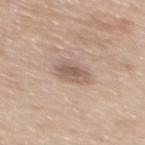Impression:
The lesion was photographed on a routine skin check and not biopsied; there is no pathology result.
Background:
Imaged with white-light lighting. Cropped from a total-body skin-imaging series; the visible field is about 15 mm. The lesion-visualizer software estimated a lesion area of about 7 mm². The lesion is on the mid back. Measured at roughly 3.5 mm in maximum diameter. A male subject, in their 50s.